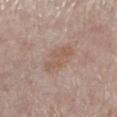This lesion was catalogued during total-body skin photography and was not selected for biopsy. The lesion is on the left lower leg. The tile uses white-light illumination. Longest diameter approximately 5 mm. A 15 mm crop from a total-body photograph taken for skin-cancer surveillance. A female patient, in their mid-50s.